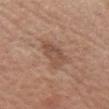| field | value |
|---|---|
| biopsy status | total-body-photography surveillance lesion; no biopsy |
| site | the head or neck |
| imaging modality | ~15 mm crop, total-body skin-cancer survey |
| lighting | white-light illumination |
| subject | female, aged around 40 |
| image-analysis metrics | a lesion color around L≈50 a*≈19 b*≈28 in CIELAB and about 8 CIELAB-L* units darker than the surrounding skin; a classifier nevus-likeness of about 5/100 and a lesion-detection confidence of about 100/100 |